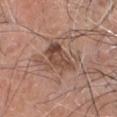Clinical impression:
Part of a total-body skin-imaging series; this lesion was reviewed on a skin check and was not flagged for biopsy.
Image and clinical context:
The total-body-photography lesion software estimated a lesion area of about 10 mm². The analysis additionally found border irregularity of about 5 on a 0–10 scale and peripheral color asymmetry of about 2. A close-up tile cropped from a whole-body skin photograph, about 15 mm across. On the head or neck. The patient is a male roughly 50 years of age.